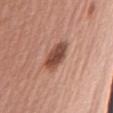This lesion was catalogued during total-body skin photography and was not selected for biopsy. The patient is a female in their 60s. A 15 mm crop from a total-body photograph taken for skin-cancer surveillance. The lesion is on the arm.